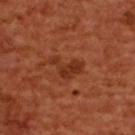This lesion was catalogued during total-body skin photography and was not selected for biopsy.
The tile uses cross-polarized illumination.
A female subject approximately 55 years of age.
The lesion is on the upper back.
Longest diameter approximately 4 mm.
A 15 mm close-up extracted from a 3D total-body photography capture.